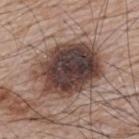notes: no biopsy performed (imaged during a skin exam)
imaging modality: 15 mm crop, total-body photography
lighting: white-light
patient: male, aged 63–67
lesion diameter: ~7.5 mm (longest diameter)
site: the upper back
automated metrics: an area of roughly 33 mm² and two-axis asymmetry of about 0.15; a mean CIELAB color near L≈39 a*≈16 b*≈20, about 19 CIELAB-L* units darker than the surrounding skin, and a normalized border contrast of about 14.5; border irregularity of about 2.5 on a 0–10 scale, internal color variation of about 7.5 on a 0–10 scale, and radial color variation of about 2; an automated nevus-likeness rating near 10 out of 100 and a lesion-detection confidence of about 100/100